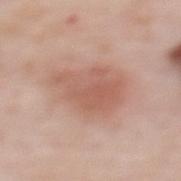Assessment: Recorded during total-body skin imaging; not selected for excision or biopsy. Acquisition and patient details: Longest diameter approximately 8 mm. A female patient, aged approximately 50. Captured under white-light illumination. The lesion is on the upper back. A close-up tile cropped from a whole-body skin photograph, about 15 mm across.